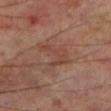Impression: Imaged during a routine full-body skin examination; the lesion was not biopsied and no histopathology is available. Clinical summary: The subject is a male aged 68–72. About 4 mm across. The tile uses cross-polarized illumination. A 15 mm close-up tile from a total-body photography series done for melanoma screening. The lesion is on the left lower leg.